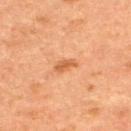Impression:
No biopsy was performed on this lesion — it was imaged during a full skin examination and was not determined to be concerning.
Acquisition and patient details:
Cropped from a whole-body photographic skin survey; the tile spans about 15 mm. A male subject aged approximately 50. The lesion is on the upper back.